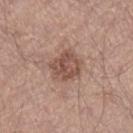Impression: Part of a total-body skin-imaging series; this lesion was reviewed on a skin check and was not flagged for biopsy. Acquisition and patient details: This is a white-light tile. A male patient, in their 40s. Located on the right lower leg. A 15 mm close-up extracted from a 3D total-body photography capture.